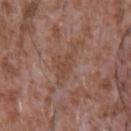A male subject, approximately 45 years of age. A 15 mm close-up tile from a total-body photography series done for melanoma screening. The lesion is on the chest.